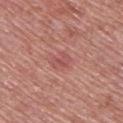Clinical impression: This lesion was catalogued during total-body skin photography and was not selected for biopsy. Context: A 15 mm close-up tile from a total-body photography series done for melanoma screening. A male patient aged approximately 50. The lesion is located on the upper back.